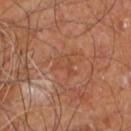From the right leg. A 15 mm close-up tile from a total-body photography series done for melanoma screening. Longest diameter approximately 3 mm. A male patient approximately 60 years of age. An algorithmic analysis of the crop reported a mean CIELAB color near L≈45 a*≈23 b*≈32 and about 5 CIELAB-L* units darker than the surrounding skin. The software also gave a nevus-likeness score of about 0/100 and a lesion-detection confidence of about 95/100. The tile uses cross-polarized illumination.